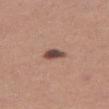Assessment: The lesion was photographed on a routine skin check and not biopsied; there is no pathology result. Image and clinical context: The lesion-visualizer software estimated a lesion area of about 4 mm², an outline eccentricity of about 0.75 (0 = round, 1 = elongated), and a symmetry-axis asymmetry near 0.25. The analysis additionally found a lesion color around L≈48 a*≈19 b*≈23 in CIELAB and a normalized border contrast of about 10.5. The lesion is on the leg. Approximately 2.5 mm at its widest. A region of skin cropped from a whole-body photographic capture, roughly 15 mm wide. A female subject in their mid-30s. Captured under white-light illumination.